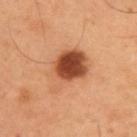Impression: The lesion was photographed on a routine skin check and not biopsied; there is no pathology result. Acquisition and patient details: The recorded lesion diameter is about 5.5 mm. A lesion tile, about 15 mm wide, cut from a 3D total-body photograph. A male subject, aged 53–57. The lesion is located on the upper back.